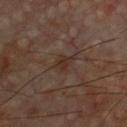subject: male, roughly 60 years of age
site: the chest
image: 15 mm crop, total-body photography